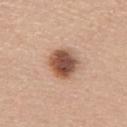{"biopsy_status": "not biopsied; imaged during a skin examination", "lesion_size": {"long_diameter_mm_approx": 4.5}, "automated_metrics": {"lesion_detection_confidence_0_100": 100}, "patient": {"sex": "female", "age_approx": 65}, "site": "mid back", "lighting": "white-light", "image": {"source": "total-body photography crop", "field_of_view_mm": 15}}